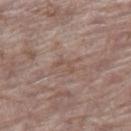notes: imaged on a skin check; not biopsied | acquisition: total-body-photography crop, ~15 mm field of view | body site: the leg | illumination: white-light illumination | subject: female, aged 83 or older | lesion size: ≈2.5 mm.